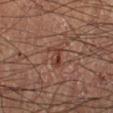Clinical impression: This lesion was catalogued during total-body skin photography and was not selected for biopsy. Background: On the left lower leg. A lesion tile, about 15 mm wide, cut from a 3D total-body photograph. The tile uses cross-polarized illumination. A male patient, aged approximately 55.